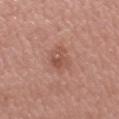Case summary:
• biopsy status: total-body-photography surveillance lesion; no biopsy
• body site: the right upper arm
• lesion diameter: about 4 mm
• automated metrics: an average lesion color of about L≈52 a*≈23 b*≈28 (CIELAB), about 8 CIELAB-L* units darker than the surrounding skin, and a lesion-to-skin contrast of about 6 (normalized; higher = more distinct); a border-irregularity index near 3.5/10 and a peripheral color-asymmetry measure near 1.5; an automated nevus-likeness rating near 50 out of 100 and a detector confidence of about 100 out of 100 that the crop contains a lesion
• patient: female, aged 68 to 72
• image: total-body-photography crop, ~15 mm field of view